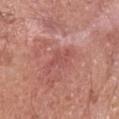Clinical impression:
Imaged during a routine full-body skin examination; the lesion was not biopsied and no histopathology is available.
Background:
A lesion tile, about 15 mm wide, cut from a 3D total-body photograph. The total-body-photography lesion software estimated an area of roughly 5.5 mm², an outline eccentricity of about 0.85 (0 = round, 1 = elongated), and a shape-asymmetry score of about 0.3 (0 = symmetric). And it measured internal color variation of about 1.5 on a 0–10 scale and radial color variation of about 0.5. The software also gave a nevus-likeness score of about 0/100 and a lesion-detection confidence of about 90/100. This is a white-light tile. About 4 mm across. A male subject, aged approximately 55. On the chest.